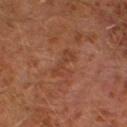Imaged during a routine full-body skin examination; the lesion was not biopsied and no histopathology is available.
The lesion is located on the left leg.
This image is a 15 mm lesion crop taken from a total-body photograph.
About 3.5 mm across.
A male patient, aged approximately 30.
Captured under cross-polarized illumination.